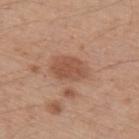follow-up = no biopsy performed (imaged during a skin exam)
lesion diameter = ≈4 mm
imaging modality = ~15 mm tile from a whole-body skin photo
site = the left upper arm
lighting = white-light
subject = male, aged around 60